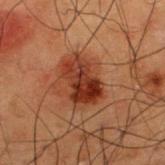Impression: Part of a total-body skin-imaging series; this lesion was reviewed on a skin check and was not flagged for biopsy. Acquisition and patient details: The total-body-photography lesion software estimated a lesion color around L≈26 a*≈23 b*≈26 in CIELAB, roughly 11 lightness units darker than nearby skin, and a normalized lesion–skin contrast near 11.5. The software also gave an automated nevus-likeness rating near 100 out of 100 and a lesion-detection confidence of about 100/100. A male patient, approximately 50 years of age. About 4.5 mm across. This is a cross-polarized tile. A region of skin cropped from a whole-body photographic capture, roughly 15 mm wide. Located on the upper back.